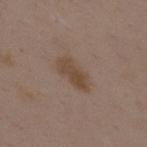Assessment: The lesion was tiled from a total-body skin photograph and was not biopsied. Image and clinical context: The lesion is located on the upper back. A region of skin cropped from a whole-body photographic capture, roughly 15 mm wide. The tile uses white-light illumination. A female patient, roughly 35 years of age.